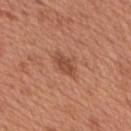Imaged with white-light lighting.
The lesion's longest dimension is about 3 mm.
The lesion is located on the upper back.
A roughly 15 mm field-of-view crop from a total-body skin photograph.
The patient is a male aged 63 to 67.
The total-body-photography lesion software estimated a border-irregularity rating of about 3/10, a color-variation rating of about 1.5/10, and a peripheral color-asymmetry measure near 0.5. And it measured a nevus-likeness score of about 60/100 and a lesion-detection confidence of about 100/100.